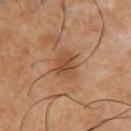The lesion was photographed on a routine skin check and not biopsied; there is no pathology result.
On the chest.
Automated tile analysis of the lesion measured an area of roughly 6.5 mm² and an outline eccentricity of about 0.5 (0 = round, 1 = elongated). The software also gave border irregularity of about 2.5 on a 0–10 scale and radial color variation of about 1. And it measured a nevus-likeness score of about 45/100 and a detector confidence of about 100 out of 100 that the crop contains a lesion.
The tile uses cross-polarized illumination.
A lesion tile, about 15 mm wide, cut from a 3D total-body photograph.
The patient is a male aged 53 to 57.